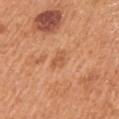Recorded during total-body skin imaging; not selected for excision or biopsy. This is a white-light tile. From the chest. This image is a 15 mm lesion crop taken from a total-body photograph. The subject is a male about 55 years old. The lesion's longest dimension is about 2.5 mm.